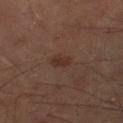Clinical summary:
Imaged with cross-polarized lighting. A male subject in their mid-50s. The lesion is located on the right lower leg. The lesion's longest dimension is about 2.5 mm. A roughly 15 mm field-of-view crop from a total-body skin photograph.